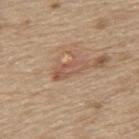<lesion>
  <biopsy_status>not biopsied; imaged during a skin examination</biopsy_status>
</lesion>